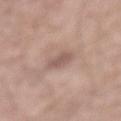Impression:
Captured during whole-body skin photography for melanoma surveillance; the lesion was not biopsied.
Acquisition and patient details:
A close-up tile cropped from a whole-body skin photograph, about 15 mm across. A male patient aged 58 to 62. Automated image analysis of the tile measured a shape-asymmetry score of about 0.2 (0 = symmetric). The analysis additionally found about 9 CIELAB-L* units darker than the surrounding skin and a normalized lesion–skin contrast near 6.5. And it measured a border-irregularity rating of about 2/10, a within-lesion color-variation index near 0.5/10, and a peripheral color-asymmetry measure near 0. It also reported a classifier nevus-likeness of about 0/100 and lesion-presence confidence of about 100/100. From the mid back. The tile uses white-light illumination.